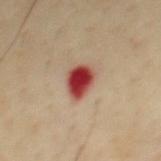| field | value |
|---|---|
| workup | total-body-photography surveillance lesion; no biopsy |
| imaging modality | 15 mm crop, total-body photography |
| site | the chest |
| subject | male, approximately 65 years of age |